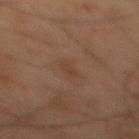The lesion was tiled from a total-body skin photograph and was not biopsied.
Cropped from a whole-body photographic skin survey; the tile spans about 15 mm.
The subject is a male aged 58 to 62.
Located on the back.